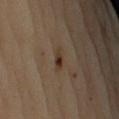follow-up = imaged on a skin check; not biopsied
acquisition = 15 mm crop, total-body photography
diameter = ≈2.5 mm
location = the left upper arm
subject = female, aged 68 to 72
automated lesion analysis = border irregularity of about 3 on a 0–10 scale and a within-lesion color-variation index near 5.5/10; a nevus-likeness score of about 95/100 and a detector confidence of about 100 out of 100 that the crop contains a lesion
illumination = cross-polarized illumination